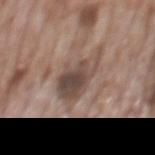This lesion was catalogued during total-body skin photography and was not selected for biopsy. Approximately 6.5 mm at its widest. A 15 mm close-up extracted from a 3D total-body photography capture. A male subject aged 68 to 72. This is a white-light tile. Located on the mid back.